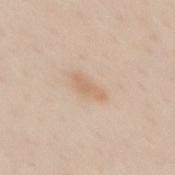{
  "biopsy_status": "not biopsied; imaged during a skin examination",
  "lighting": "white-light",
  "lesion_size": {
    "long_diameter_mm_approx": 3.5
  },
  "site": "back",
  "image": {
    "source": "total-body photography crop",
    "field_of_view_mm": 15
  },
  "automated_metrics": {
    "cielab_L": 66,
    "cielab_a": 17,
    "cielab_b": 32,
    "vs_skin_contrast_norm": 5.5,
    "color_variation_0_10": 0.5,
    "peripheral_color_asymmetry": 0.0
  },
  "patient": {
    "sex": "female",
    "age_approx": 30
  }
}